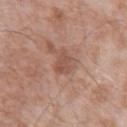notes: no biopsy performed (imaged during a skin exam) | image: ~15 mm tile from a whole-body skin photo | anatomic site: the arm | subject: male, aged around 75.